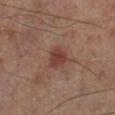This lesion was catalogued during total-body skin photography and was not selected for biopsy. From the right lower leg. About 2.5 mm across. Imaged with cross-polarized lighting. A male subject, in their mid- to late 60s. A lesion tile, about 15 mm wide, cut from a 3D total-body photograph.